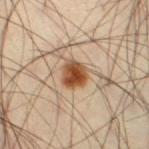Part of a total-body skin-imaging series; this lesion was reviewed on a skin check and was not flagged for biopsy.
The subject is a male aged 38 to 42.
A 15 mm crop from a total-body photograph taken for skin-cancer surveillance.
The lesion is located on the leg.